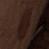Findings:
* location — the abdomen
* image — ~15 mm crop, total-body skin-cancer survey
* patient — male, approximately 70 years of age
* diameter — ~8 mm (longest diameter)
* TBP lesion metrics — an area of roughly 27 mm², a shape eccentricity near 0.8, and two-axis asymmetry of about 0.15; a peripheral color-asymmetry measure near 1; an automated nevus-likeness rating near 10 out of 100 and lesion-presence confidence of about 100/100